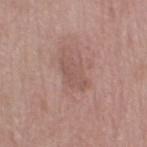Case summary:
• notes: total-body-photography surveillance lesion; no biopsy
• location: the left thigh
• acquisition: total-body-photography crop, ~15 mm field of view
• subject: female, aged approximately 70